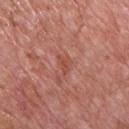– follow-up — catalogued during a skin exam; not biopsied
– location — the chest
– illumination — white-light illumination
– patient — male, aged 73–77
– image — ~15 mm crop, total-body skin-cancer survey
– diameter — ≈2.5 mm